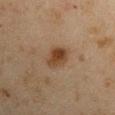About 3.5 mm across.
The lesion is on the right upper arm.
The patient is a male in their mid-40s.
Cropped from a total-body skin-imaging series; the visible field is about 15 mm.
Captured under cross-polarized illumination.
An algorithmic analysis of the crop reported a lesion area of about 6.5 mm² and two-axis asymmetry of about 0.15. It also reported a lesion color around L≈34 a*≈15 b*≈27 in CIELAB, about 9 CIELAB-L* units darker than the surrounding skin, and a normalized lesion–skin contrast near 9. The software also gave a border-irregularity index near 1.5/10, a color-variation rating of about 5/10, and peripheral color asymmetry of about 2.